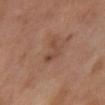* follow-up — catalogued during a skin exam; not biopsied
* body site — the abdomen
* TBP lesion metrics — a mean CIELAB color near L≈46 a*≈20 b*≈28 and a normalized border contrast of about 5.5; a nevus-likeness score of about 0/100
* lesion diameter — ≈3 mm
* image — ~15 mm crop, total-body skin-cancer survey
* tile lighting — cross-polarized illumination
* subject — female, in their mid-50s